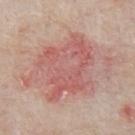Captured during whole-body skin photography for melanoma surveillance; the lesion was not biopsied.
The total-body-photography lesion software estimated a classifier nevus-likeness of about 5/100 and a lesion-detection confidence of about 95/100.
Located on the chest.
A 15 mm close-up tile from a total-body photography series done for melanoma screening.
The subject is a male in their mid-60s.
The recorded lesion diameter is about 7.5 mm.